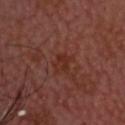Findings:
– biopsy status · catalogued during a skin exam; not biopsied
– automated lesion analysis · an area of roughly 4 mm², an eccentricity of roughly 0.7, and two-axis asymmetry of about 0.3; an average lesion color of about L≈30 a*≈24 b*≈27 (CIELAB), about 5 CIELAB-L* units darker than the surrounding skin, and a normalized lesion–skin contrast near 6.5; a nevus-likeness score of about 0/100 and lesion-presence confidence of about 100/100
– diameter · ≈2.5 mm
– location · the head or neck
– imaging modality · total-body-photography crop, ~15 mm field of view
– subject · male, aged around 65
– lighting · cross-polarized illumination